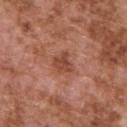This lesion was catalogued during total-body skin photography and was not selected for biopsy. Automated image analysis of the tile measured a border-irregularity index near 3/10 and peripheral color asymmetry of about 1. The recorded lesion diameter is about 2.5 mm. The lesion is on the upper back. A male patient, in their mid-70s. A region of skin cropped from a whole-body photographic capture, roughly 15 mm wide.